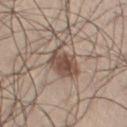Q: Is there a histopathology result?
A: catalogued during a skin exam; not biopsied
Q: Lesion location?
A: the leg
Q: How was this image acquired?
A: ~15 mm crop, total-body skin-cancer survey
Q: Illumination type?
A: white-light illumination
Q: Patient demographics?
A: male, in their mid-50s
Q: What is the lesion's diameter?
A: ~4 mm (longest diameter)